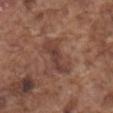Q: Was a biopsy performed?
A: imaged on a skin check; not biopsied
Q: What lighting was used for the tile?
A: white-light
Q: What did automated image analysis measure?
A: a lesion color around L≈41 a*≈20 b*≈25 in CIELAB, roughly 8 lightness units darker than nearby skin, and a lesion-to-skin contrast of about 6.5 (normalized; higher = more distinct); a border-irregularity rating of about 4/10, internal color variation of about 3 on a 0–10 scale, and peripheral color asymmetry of about 1; lesion-presence confidence of about 85/100
Q: Lesion location?
A: the mid back
Q: Lesion size?
A: ~5.5 mm (longest diameter)
Q: What is the imaging modality?
A: 15 mm crop, total-body photography
Q: Who is the patient?
A: male, about 75 years old